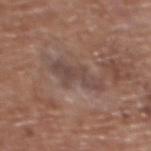Q: Was a biopsy performed?
A: imaged on a skin check; not biopsied
Q: Lesion location?
A: the upper back
Q: What kind of image is this?
A: ~15 mm crop, total-body skin-cancer survey
Q: What lighting was used for the tile?
A: white-light
Q: Patient demographics?
A: female, aged 73 to 77
Q: What is the lesion's diameter?
A: ~5.5 mm (longest diameter)